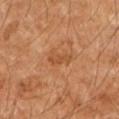Case summary:
* notes — catalogued during a skin exam; not biopsied
* automated lesion analysis — about 7 CIELAB-L* units darker than the surrounding skin and a lesion-to-skin contrast of about 5.5 (normalized; higher = more distinct)
* location — the arm
* size — ≈3 mm
* illumination — cross-polarized
* acquisition — ~15 mm crop, total-body skin-cancer survey
* subject — male, aged around 55